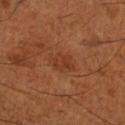{
  "biopsy_status": "not biopsied; imaged during a skin examination",
  "lighting": "cross-polarized",
  "image": {
    "source": "total-body photography crop",
    "field_of_view_mm": 15
  },
  "site": "left lower leg",
  "patient": {
    "sex": "male",
    "age_approx": 65
  }
}